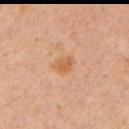Clinical impression:
This lesion was catalogued during total-body skin photography and was not selected for biopsy.
Context:
This is a cross-polarized tile. Automated tile analysis of the lesion measured a color-variation rating of about 2/10 and a peripheral color-asymmetry measure near 0.5. It also reported lesion-presence confidence of about 100/100. From the left upper arm. The recorded lesion diameter is about 2.5 mm. A lesion tile, about 15 mm wide, cut from a 3D total-body photograph. The patient is a male in their mid-40s.